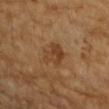notes: no biopsy performed (imaged during a skin exam)
lighting: cross-polarized illumination
anatomic site: the right upper arm
subject: female, aged around 70
imaging modality: ~15 mm crop, total-body skin-cancer survey
diameter: ~3.5 mm (longest diameter)